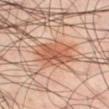Assessment:
Imaged during a routine full-body skin examination; the lesion was not biopsied and no histopathology is available.
Background:
A male subject, aged 38 to 42. From the left thigh. An algorithmic analysis of the crop reported a border-irregularity rating of about 2.5/10, internal color variation of about 5 on a 0–10 scale, and a peripheral color-asymmetry measure near 1.5. The analysis additionally found an automated nevus-likeness rating near 95 out of 100 and lesion-presence confidence of about 100/100. A 15 mm close-up tile from a total-body photography series done for melanoma screening. Imaged with cross-polarized lighting.